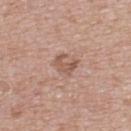- follow-up: no biopsy performed (imaged during a skin exam)
- patient: female, about 40 years old
- body site: the upper back
- image: ~15 mm crop, total-body skin-cancer survey